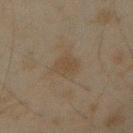Assessment: This lesion was catalogued during total-body skin photography and was not selected for biopsy. Acquisition and patient details: About 3 mm across. A male subject roughly 45 years of age. From the right forearm. Cropped from a total-body skin-imaging series; the visible field is about 15 mm. This is a cross-polarized tile.